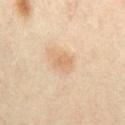Q: Is there a histopathology result?
A: catalogued during a skin exam; not biopsied
Q: What lighting was used for the tile?
A: cross-polarized illumination
Q: Lesion size?
A: ~3 mm (longest diameter)
Q: What are the patient's age and sex?
A: male, approximately 55 years of age
Q: What is the imaging modality?
A: ~15 mm crop, total-body skin-cancer survey
Q: Where on the body is the lesion?
A: the chest